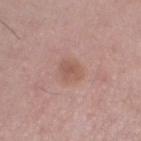Q: Was a biopsy performed?
A: imaged on a skin check; not biopsied
Q: How was this image acquired?
A: ~15 mm tile from a whole-body skin photo
Q: What lighting was used for the tile?
A: white-light
Q: Lesion size?
A: ~2.5 mm (longest diameter)
Q: Who is the patient?
A: female, aged 48 to 52
Q: Where on the body is the lesion?
A: the left thigh